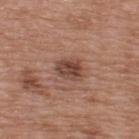| feature | finding |
|---|---|
| follow-up | imaged on a skin check; not biopsied |
| illumination | white-light |
| size | about 3 mm |
| subject | male, in their 50s |
| automated lesion analysis | an average lesion color of about L≈44 a*≈20 b*≈26 (CIELAB), a lesion–skin lightness drop of about 11, and a normalized border contrast of about 8.5 |
| acquisition | total-body-photography crop, ~15 mm field of view |
| body site | the upper back |